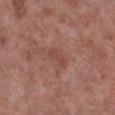<lesion>
  <biopsy_status>not biopsied; imaged during a skin examination</biopsy_status>
  <site>right lower leg</site>
  <patient>
    <sex>male</sex>
    <age_approx>55</age_approx>
  </patient>
  <image>
    <source>total-body photography crop</source>
    <field_of_view_mm>15</field_of_view_mm>
  </image>
</lesion>